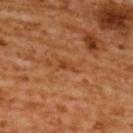• notes · no biopsy performed (imaged during a skin exam)
• size · about 2 mm
• site · the upper back
• subject · female, about 55 years old
• TBP lesion metrics · border irregularity of about 3.5 on a 0–10 scale, a color-variation rating of about 0/10, and peripheral color asymmetry of about 0; a classifier nevus-likeness of about 0/100
• image source · ~15 mm crop, total-body skin-cancer survey